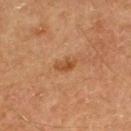Clinical impression:
This lesion was catalogued during total-body skin photography and was not selected for biopsy.
Acquisition and patient details:
Cropped from a total-body skin-imaging series; the visible field is about 15 mm. A male subject aged around 60.